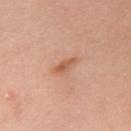Assessment:
No biopsy was performed on this lesion — it was imaged during a full skin examination and was not determined to be concerning.
Background:
Approximately 3 mm at its widest. The lesion is on the chest. Captured under white-light illumination. The patient is a female roughly 50 years of age. A 15 mm close-up tile from a total-body photography series done for melanoma screening.